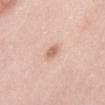Impression:
The lesion was tiled from a total-body skin photograph and was not biopsied.
Acquisition and patient details:
The tile uses white-light illumination. A lesion tile, about 15 mm wide, cut from a 3D total-body photograph. A female subject, aged 28 to 32. The lesion is on the abdomen. Measured at roughly 2.5 mm in maximum diameter.